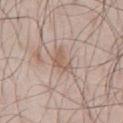Acquisition and patient details:
Approximately 3 mm at its widest. Cropped from a whole-body photographic skin survey; the tile spans about 15 mm. The subject is a male aged approximately 45. The lesion is located on the abdomen. Imaged with white-light lighting.